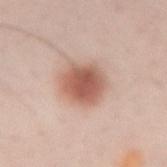Image and clinical context:
A female subject, aged 38 to 42. From the right forearm. This is a white-light tile. Cropped from a total-body skin-imaging series; the visible field is about 15 mm. Longest diameter approximately 5 mm. The lesion-visualizer software estimated a lesion color around L≈58 a*≈23 b*≈28 in CIELAB, roughly 14 lightness units darker than nearby skin, and a normalized lesion–skin contrast near 9. The software also gave a border-irregularity rating of about 1.5/10 and peripheral color asymmetry of about 1.